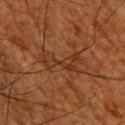{"biopsy_status": "not biopsied; imaged during a skin examination", "image": {"source": "total-body photography crop", "field_of_view_mm": 15}, "site": "upper back", "lesion_size": {"long_diameter_mm_approx": 5.0}, "patient": {"sex": "male", "age_approx": 65}}